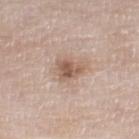| field | value |
|---|---|
| notes | total-body-photography surveillance lesion; no biopsy |
| imaging modality | 15 mm crop, total-body photography |
| location | the right lower leg |
| lighting | white-light |
| diameter | ~3 mm (longest diameter) |
| patient | male, aged approximately 80 |